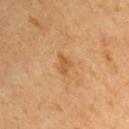Impression: Captured during whole-body skin photography for melanoma surveillance; the lesion was not biopsied. Clinical summary: The tile uses cross-polarized illumination. A male patient, aged 63 to 67. A 15 mm crop from a total-body photograph taken for skin-cancer surveillance. The lesion is on the left arm. Approximately 2.5 mm at its widest. The total-body-photography lesion software estimated an area of roughly 3 mm². It also reported a nevus-likeness score of about 0/100 and a detector confidence of about 100 out of 100 that the crop contains a lesion.